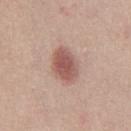No biopsy was performed on this lesion — it was imaged during a full skin examination and was not determined to be concerning. Located on the leg. This is a white-light tile. A 15 mm close-up extracted from a 3D total-body photography capture. A female patient about 20 years old. Longest diameter approximately 4 mm. Automated image analysis of the tile measured a lesion area of about 9.5 mm², an eccentricity of roughly 0.7, and two-axis asymmetry of about 0.15. It also reported a mean CIELAB color near L≈55 a*≈22 b*≈24, roughly 13 lightness units darker than nearby skin, and a normalized lesion–skin contrast near 8.5. It also reported an automated nevus-likeness rating near 100 out of 100 and a detector confidence of about 100 out of 100 that the crop contains a lesion.